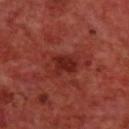<tbp_lesion>
  <biopsy_status>not biopsied; imaged during a skin examination</biopsy_status>
  <lesion_size>
    <long_diameter_mm_approx>3.0</long_diameter_mm_approx>
  </lesion_size>
  <patient>
    <sex>male</sex>
    <age_approx>70</age_approx>
  </patient>
  <lighting>cross-polarized</lighting>
  <image>
    <source>total-body photography crop</source>
    <field_of_view_mm>15</field_of_view_mm>
  </image>
  <site>upper back</site>
</tbp_lesion>